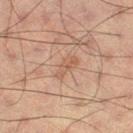{"biopsy_status": "not biopsied; imaged during a skin examination", "image": {"source": "total-body photography crop", "field_of_view_mm": 15}, "lighting": "cross-polarized", "patient": {"sex": "male", "age_approx": 60}, "lesion_size": {"long_diameter_mm_approx": 3.0}}